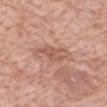Assessment:
No biopsy was performed on this lesion — it was imaged during a full skin examination and was not determined to be concerning.
Acquisition and patient details:
The subject is a female in their 70s. Longest diameter approximately 4 mm. On the arm. A roughly 15 mm field-of-view crop from a total-body skin photograph.